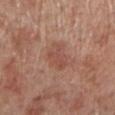{"biopsy_status": "not biopsied; imaged during a skin examination", "lesion_size": {"long_diameter_mm_approx": 3.5}, "lighting": "white-light", "image": {"source": "total-body photography crop", "field_of_view_mm": 15}, "site": "left lower leg", "patient": {"sex": "male", "age_approx": 70}}